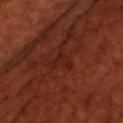Findings:
- biopsy status — total-body-photography surveillance lesion; no biopsy
- patient — male, aged 48 to 52
- TBP lesion metrics — a footprint of about 4 mm², a shape eccentricity near 0.5, and two-axis asymmetry of about 0.55; border irregularity of about 6.5 on a 0–10 scale and radial color variation of about 0
- lighting — cross-polarized
- imaging modality — ~15 mm tile from a whole-body skin photo
- location — the head or neck